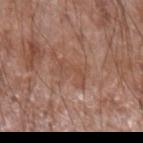Recorded during total-body skin imaging; not selected for excision or biopsy.
Longest diameter approximately 3.5 mm.
On the left forearm.
A male patient, aged 58–62.
A region of skin cropped from a whole-body photographic capture, roughly 15 mm wide.
The tile uses white-light illumination.